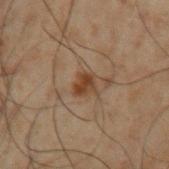Imaged during a routine full-body skin examination; the lesion was not biopsied and no histopathology is available. On the left upper arm. Captured under cross-polarized illumination. A 15 mm crop from a total-body photograph taken for skin-cancer surveillance. Longest diameter approximately 2.5 mm. The subject is a male aged approximately 50.